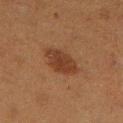Part of a total-body skin-imaging series; this lesion was reviewed on a skin check and was not flagged for biopsy. On the right lower leg. Approximately 4.5 mm at its widest. A 15 mm close-up tile from a total-body photography series done for melanoma screening. The subject is a female aged around 40. The tile uses cross-polarized illumination.